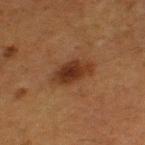This lesion was catalogued during total-body skin photography and was not selected for biopsy.
The lesion is located on the left thigh.
A female subject approximately 50 years of age.
A 15 mm crop from a total-body photograph taken for skin-cancer surveillance.